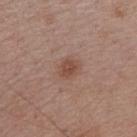Part of a total-body skin-imaging series; this lesion was reviewed on a skin check and was not flagged for biopsy. A female subject, aged approximately 55. Cropped from a total-body skin-imaging series; the visible field is about 15 mm. Located on the right upper arm. An algorithmic analysis of the crop reported an average lesion color of about L≈47 a*≈20 b*≈27 (CIELAB) and about 8 CIELAB-L* units darker than the surrounding skin. And it measured a classifier nevus-likeness of about 75/100 and lesion-presence confidence of about 100/100. About 2.5 mm across. Imaged with white-light lighting.